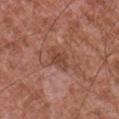The lesion was photographed on a routine skin check and not biopsied; there is no pathology result. The lesion is located on the chest. A lesion tile, about 15 mm wide, cut from a 3D total-body photograph. Approximately 3 mm at its widest. Automated image analysis of the tile measured a shape-asymmetry score of about 0.3 (0 = symmetric). And it measured an automated nevus-likeness rating near 0 out of 100 and a detector confidence of about 100 out of 100 that the crop contains a lesion. This is a white-light tile. A male subject, in their mid-40s.